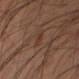workup = catalogued during a skin exam; not biopsied | acquisition = ~15 mm tile from a whole-body skin photo | lesion size = ~3 mm (longest diameter) | subject = male, aged 53–57 | illumination = cross-polarized | body site = the arm.